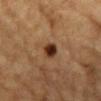Assessment: No biopsy was performed on this lesion — it was imaged during a full skin examination and was not determined to be concerning. Acquisition and patient details: A 15 mm crop from a total-body photograph taken for skin-cancer surveillance. From the front of the torso. The recorded lesion diameter is about 2.5 mm. Captured under cross-polarized illumination. The patient is a male roughly 85 years of age or older.